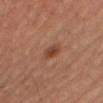Captured during whole-body skin photography for melanoma surveillance; the lesion was not biopsied.
The tile uses cross-polarized illumination.
The lesion-visualizer software estimated roughly 9 lightness units darker than nearby skin and a normalized border contrast of about 7.5. The software also gave a border-irregularity rating of about 1.5/10 and a within-lesion color-variation index near 3.5/10.
On the front of the torso.
A male subject aged 33 to 37.
Cropped from a total-body skin-imaging series; the visible field is about 15 mm.
Measured at roughly 2.5 mm in maximum diameter.